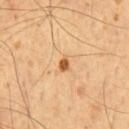Case summary:
• biopsy status · no biopsy performed (imaged during a skin exam)
• location · the chest
• patient · male, in their mid-50s
• image source · ~15 mm tile from a whole-body skin photo
• tile lighting · cross-polarized
• size · ≈2 mm